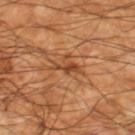Assessment:
The lesion was tiled from a total-body skin photograph and was not biopsied.
Context:
Cropped from a total-body skin-imaging series; the visible field is about 15 mm. The lesion's longest dimension is about 3 mm. The lesion is located on the right lower leg. This is a cross-polarized tile. The patient is a male about 60 years old.